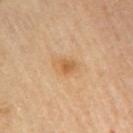Q: Was this lesion biopsied?
A: imaged on a skin check; not biopsied
Q: How was the tile lit?
A: cross-polarized
Q: What is the lesion's diameter?
A: ≈3 mm
Q: Who is the patient?
A: male, aged 83 or over
Q: Where on the body is the lesion?
A: the right upper arm
Q: What is the imaging modality?
A: ~15 mm crop, total-body skin-cancer survey